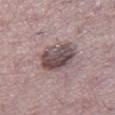Clinical impression:
The lesion was tiled from a total-body skin photograph and was not biopsied.
Context:
The tile uses white-light illumination. The recorded lesion diameter is about 4 mm. A male patient, aged approximately 55. Located on the right thigh. Automated tile analysis of the lesion measured an average lesion color of about L≈47 a*≈15 b*≈16 (CIELAB) and a normalized lesion–skin contrast near 10. It also reported a classifier nevus-likeness of about 25/100 and lesion-presence confidence of about 95/100. A 15 mm crop from a total-body photograph taken for skin-cancer surveillance.